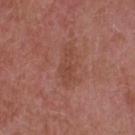workup: no biopsy performed (imaged during a skin exam); tile lighting: white-light; patient: female, aged approximately 60; location: the upper back; lesion size: ~4 mm (longest diameter); imaging modality: ~15 mm crop, total-body skin-cancer survey.